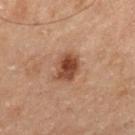notes = imaged on a skin check; not biopsied
anatomic site = the left thigh
patient = male, roughly 70 years of age
image = 15 mm crop, total-body photography